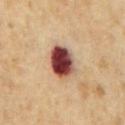follow-up = imaged on a skin check; not biopsied